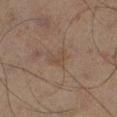This lesion was catalogued during total-body skin photography and was not selected for biopsy. A male patient, about 65 years old. The lesion is on the left lower leg. A 15 mm close-up tile from a total-body photography series done for melanoma screening.